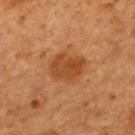workup — no biopsy performed (imaged during a skin exam)
patient — male, aged 63–67
tile lighting — cross-polarized illumination
site — the upper back
lesion size — ~4 mm (longest diameter)
imaging modality — ~15 mm crop, total-body skin-cancer survey